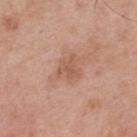Notes:
* body site: the upper back
* acquisition: total-body-photography crop, ~15 mm field of view
* subject: male, aged 53 to 57